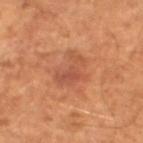Q: Was this lesion biopsied?
A: total-body-photography surveillance lesion; no biopsy
Q: Lesion size?
A: about 4 mm
Q: How was the tile lit?
A: cross-polarized illumination
Q: Automated lesion metrics?
A: an area of roughly 8 mm², a shape eccentricity near 0.75, and a shape-asymmetry score of about 0.5 (0 = symmetric); a nevus-likeness score of about 10/100 and a lesion-detection confidence of about 100/100
Q: Who is the patient?
A: male, aged approximately 65
Q: What is the imaging modality?
A: total-body-photography crop, ~15 mm field of view